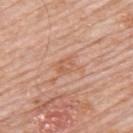  biopsy_status: not biopsied; imaged during a skin examination
  automated_metrics:
    cielab_L: 60
    cielab_a: 23
    cielab_b: 33
    vs_skin_darker_L: 6.0
    border_irregularity_0_10: 3.0
    color_variation_0_10: 2.5
    peripheral_color_asymmetry: 0.5
    nevus_likeness_0_100: 0
  site: upper back
  lighting: white-light
  patient:
    sex: male
    age_approx: 80
  lesion_size:
    long_diameter_mm_approx: 3.0
  image:
    source: total-body photography crop
    field_of_view_mm: 15